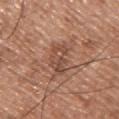| feature | finding |
|---|---|
| follow-up | no biopsy performed (imaged during a skin exam) |
| illumination | white-light illumination |
| automated lesion analysis | an average lesion color of about L≈49 a*≈21 b*≈28 (CIELAB) and a lesion-to-skin contrast of about 6 (normalized; higher = more distinct); a border-irregularity index near 4/10, a within-lesion color-variation index near 5/10, and peripheral color asymmetry of about 2; an automated nevus-likeness rating near 5 out of 100 and a lesion-detection confidence of about 100/100 |
| subject | male, aged around 50 |
| lesion diameter | about 4 mm |
| image source | ~15 mm crop, total-body skin-cancer survey |
| anatomic site | the upper back |